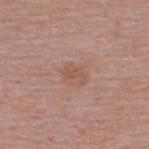Q: Was this lesion biopsied?
A: total-body-photography surveillance lesion; no biopsy
Q: Lesion size?
A: ~2.5 mm (longest diameter)
Q: Who is the patient?
A: male, aged approximately 40
Q: What kind of image is this?
A: ~15 mm tile from a whole-body skin photo
Q: What did automated image analysis measure?
A: an average lesion color of about L≈53 a*≈21 b*≈28 (CIELAB), about 6 CIELAB-L* units darker than the surrounding skin, and a lesion-to-skin contrast of about 5.5 (normalized; higher = more distinct)
Q: Lesion location?
A: the back
Q: Illumination type?
A: white-light illumination